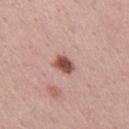Captured during whole-body skin photography for melanoma surveillance; the lesion was not biopsied. A male patient, approximately 50 years of age. From the right upper arm. Cropped from a whole-body photographic skin survey; the tile spans about 15 mm. Captured under white-light illumination. The recorded lesion diameter is about 3 mm.